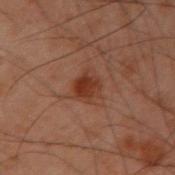This image is a 15 mm lesion crop taken from a total-body photograph.
Located on the left arm.
A male patient in their 50s.
Automated image analysis of the tile measured a lesion area of about 5.5 mm², an outline eccentricity of about 0.25 (0 = round, 1 = elongated), and a symmetry-axis asymmetry near 0.15. It also reported a nevus-likeness score of about 90/100 and lesion-presence confidence of about 100/100.
The tile uses cross-polarized illumination.
The recorded lesion diameter is about 2.5 mm.